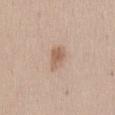No biopsy was performed on this lesion — it was imaged during a full skin examination and was not determined to be concerning.
This is a white-light tile.
This image is a 15 mm lesion crop taken from a total-body photograph.
The lesion's longest dimension is about 2.5 mm.
The lesion is located on the abdomen.
The subject is a female approximately 40 years of age.